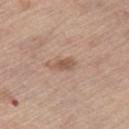  biopsy_status: not biopsied; imaged during a skin examination
  lighting: white-light
  image:
    source: total-body photography crop
    field_of_view_mm: 15
  patient:
    sex: male
    age_approx: 70
  automated_metrics:
    nevus_likeness_0_100: 5
    lesion_detection_confidence_0_100: 100
  lesion_size:
    long_diameter_mm_approx: 3.0
  site: right thigh